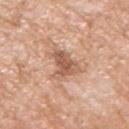No biopsy was performed on this lesion — it was imaged during a full skin examination and was not determined to be concerning. Approximately 4.5 mm at its widest. A male patient aged approximately 60. Cropped from a whole-body photographic skin survey; the tile spans about 15 mm. The lesion is on the right upper arm.